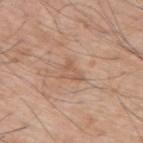| feature | finding |
|---|---|
| notes | no biopsy performed (imaged during a skin exam) |
| anatomic site | the upper back |
| lighting | white-light illumination |
| image | ~15 mm tile from a whole-body skin photo |
| lesion size | ≈3 mm |
| patient | male, aged 68 to 72 |